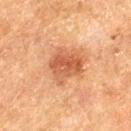No biopsy was performed on this lesion — it was imaged during a full skin examination and was not determined to be concerning. The lesion-visualizer software estimated an eccentricity of roughly 0.35 and a shape-asymmetry score of about 0.3 (0 = symmetric). The software also gave an average lesion color of about L≈46 a*≈23 b*≈32 (CIELAB), about 10 CIELAB-L* units darker than the surrounding skin, and a normalized border contrast of about 7.5. The software also gave a nevus-likeness score of about 55/100. A region of skin cropped from a whole-body photographic capture, roughly 15 mm wide. The patient is a male aged 73–77. From the left thigh. Longest diameter approximately 4 mm. The tile uses cross-polarized illumination.